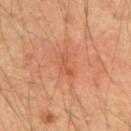Clinical impression:
Captured during whole-body skin photography for melanoma surveillance; the lesion was not biopsied.
Context:
Captured under cross-polarized illumination. On the upper back. A male patient, aged approximately 55. Longest diameter approximately 3 mm. A region of skin cropped from a whole-body photographic capture, roughly 15 mm wide.